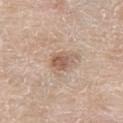Q: What are the patient's age and sex?
A: male, approximately 80 years of age
Q: How was this image acquired?
A: 15 mm crop, total-body photography
Q: Lesion size?
A: about 2.5 mm
Q: What is the anatomic site?
A: the leg